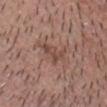workup: imaged on a skin check; not biopsied | size: about 4 mm | patient: male, aged 38–42 | site: the head or neck | image: ~15 mm crop, total-body skin-cancer survey | TBP lesion metrics: a footprint of about 6.5 mm², a shape eccentricity near 0.8, and a shape-asymmetry score of about 0.5 (0 = symmetric); a lesion–skin lightness drop of about 8 and a normalized lesion–skin contrast near 6; a border-irregularity index near 6/10, a color-variation rating of about 3/10, and a peripheral color-asymmetry measure near 1; an automated nevus-likeness rating near 0 out of 100 and a detector confidence of about 85 out of 100 that the crop contains a lesion | tile lighting: white-light.